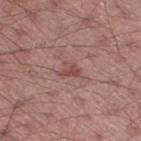Imaged during a routine full-body skin examination; the lesion was not biopsied and no histopathology is available. A region of skin cropped from a whole-body photographic capture, roughly 15 mm wide. The recorded lesion diameter is about 2.5 mm. The lesion is located on the left thigh. Captured under white-light illumination. A male patient aged 43–47.